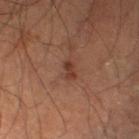Q: Was a biopsy performed?
A: catalogued during a skin exam; not biopsied
Q: What kind of image is this?
A: ~15 mm tile from a whole-body skin photo
Q: Patient demographics?
A: male, about 60 years old
Q: What is the lesion's diameter?
A: about 2 mm
Q: How was the tile lit?
A: cross-polarized illumination
Q: What is the anatomic site?
A: the left thigh